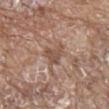biopsy status=no biopsy performed (imaged during a skin exam); subject=male, approximately 80 years of age; lesion diameter=about 3 mm; site=the back; image source=total-body-photography crop, ~15 mm field of view.